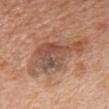Case summary:
– notes: imaged on a skin check; not biopsied
– subject: male, aged 63 to 67
– anatomic site: the chest
– size: about 10 mm
– image source: ~15 mm tile from a whole-body skin photo